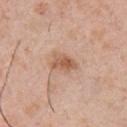No biopsy was performed on this lesion — it was imaged during a full skin examination and was not determined to be concerning.
Cropped from a whole-body photographic skin survey; the tile spans about 15 mm.
An algorithmic analysis of the crop reported a lesion color around L≈58 a*≈21 b*≈32 in CIELAB, a lesion–skin lightness drop of about 10, and a normalized lesion–skin contrast near 7.5. The analysis additionally found border irregularity of about 3 on a 0–10 scale and a color-variation rating of about 4/10. And it measured a classifier nevus-likeness of about 60/100 and a detector confidence of about 100 out of 100 that the crop contains a lesion.
The patient is a male aged 28 to 32.
Approximately 3 mm at its widest.
The lesion is on the chest.